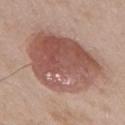Clinical impression: Part of a total-body skin-imaging series; this lesion was reviewed on a skin check and was not flagged for biopsy. Clinical summary: The tile uses white-light illumination. An algorithmic analysis of the crop reported an area of roughly 48 mm², a shape eccentricity near 0.5, and a shape-asymmetry score of about 0.2 (0 = symmetric). The analysis additionally found a border-irregularity index near 2/10, internal color variation of about 7 on a 0–10 scale, and peripheral color asymmetry of about 2.5. It also reported a classifier nevus-likeness of about 100/100 and a detector confidence of about 100 out of 100 that the crop contains a lesion. A region of skin cropped from a whole-body photographic capture, roughly 15 mm wide. The lesion is located on the right upper arm. A male patient in their mid- to late 50s. The lesion's longest dimension is about 8.5 mm.